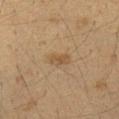workup = imaged on a skin check; not biopsied
lesion size = about 2.5 mm
acquisition = total-body-photography crop, ~15 mm field of view
illumination = cross-polarized illumination
body site = the left upper arm
patient = female, in their 40s